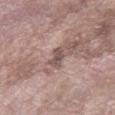notes = catalogued during a skin exam; not biopsied
patient = male, aged around 60
lighting = white-light illumination
acquisition = ~15 mm crop, total-body skin-cancer survey
anatomic site = the left forearm
lesion diameter = ~2.5 mm (longest diameter)